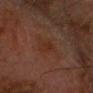notes = no biopsy performed (imaged during a skin exam) | image source = 15 mm crop, total-body photography | tile lighting = cross-polarized illumination | body site = the chest | subject = male, approximately 75 years of age | lesion diameter = about 3 mm.